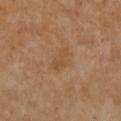No biopsy was performed on this lesion — it was imaged during a full skin examination and was not determined to be concerning.
The lesion is located on the chest.
Cropped from a whole-body photographic skin survey; the tile spans about 15 mm.
Captured under cross-polarized illumination.
A female patient, about 55 years old.
The recorded lesion diameter is about 3.5 mm.